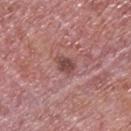biopsy status=no biopsy performed (imaged during a skin exam).